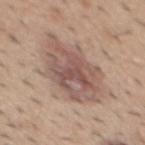follow-up — total-body-photography surveillance lesion; no biopsy
patient — male, aged 38 to 42
imaging modality — ~15 mm crop, total-body skin-cancer survey
location — the mid back